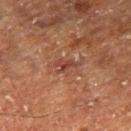Recorded during total-body skin imaging; not selected for excision or biopsy.
From the leg.
Captured under cross-polarized illumination.
A 15 mm close-up tile from a total-body photography series done for melanoma screening.
A male subject about 75 years old.
About 2.5 mm across.
Automated tile analysis of the lesion measured an average lesion color of about L≈33 a*≈21 b*≈23 (CIELAB), a lesion–skin lightness drop of about 7, and a normalized lesion–skin contrast near 7.